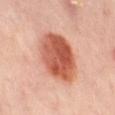The lesion was photographed on a routine skin check and not biopsied; there is no pathology result. A female patient aged 48 to 52. Automated image analysis of the tile measured border irregularity of about 1 on a 0–10 scale, a within-lesion color-variation index near 7.5/10, and radial color variation of about 2.5. From the left thigh. A 15 mm crop from a total-body photograph taken for skin-cancer surveillance. This is a cross-polarized tile. Approximately 7 mm at its widest.